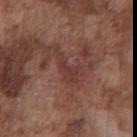From the chest. An algorithmic analysis of the crop reported a lesion area of about 6.5 mm², an eccentricity of roughly 0.9, and a symmetry-axis asymmetry near 0.55. The software also gave a border-irregularity rating of about 7/10, a within-lesion color-variation index near 2/10, and peripheral color asymmetry of about 0.5. The subject is a male in their mid- to late 70s. This is a white-light tile. This image is a 15 mm lesion crop taken from a total-body photograph. Approximately 5 mm at its widest.